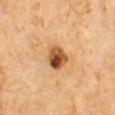Part of a total-body skin-imaging series; this lesion was reviewed on a skin check and was not flagged for biopsy. Longest diameter approximately 3.5 mm. On the upper back. Automated tile analysis of the lesion measured an automated nevus-likeness rating near 85 out of 100 and a detector confidence of about 100 out of 100 that the crop contains a lesion. A 15 mm close-up tile from a total-body photography series done for melanoma screening. Imaged with cross-polarized lighting. A male patient about 60 years old.